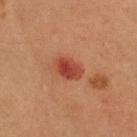biopsy status = total-body-photography surveillance lesion; no biopsy
acquisition = ~15 mm crop, total-body skin-cancer survey
anatomic site = the head or neck
patient = male, roughly 35 years of age